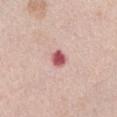site: the front of the torso; subject: female, approximately 65 years of age; imaging modality: ~15 mm crop, total-body skin-cancer survey.